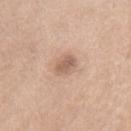Imaged during a routine full-body skin examination; the lesion was not biopsied and no histopathology is available.
Longest diameter approximately 3 mm.
A female subject roughly 75 years of age.
On the mid back.
Imaged with white-light lighting.
A roughly 15 mm field-of-view crop from a total-body skin photograph.